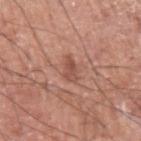Findings:
- follow-up: total-body-photography surveillance lesion; no biopsy
- lesion size: ~2.5 mm (longest diameter)
- image: ~15 mm crop, total-body skin-cancer survey
- automated metrics: an area of roughly 3 mm², an eccentricity of roughly 0.85, and a symmetry-axis asymmetry near 0.35; border irregularity of about 4 on a 0–10 scale, a color-variation rating of about 2/10, and peripheral color asymmetry of about 0
- subject: male, aged around 75
- body site: the left upper arm
- illumination: white-light illumination